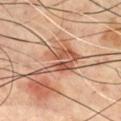Findings:
* size · ~6.5 mm (longest diameter)
* image source · ~15 mm crop, total-body skin-cancer survey
* tile lighting · cross-polarized
* location · the front of the torso
* subject · male, about 70 years old